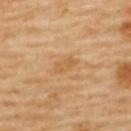Q: Was this lesion biopsied?
A: catalogued during a skin exam; not biopsied
Q: Automated lesion metrics?
A: a classifier nevus-likeness of about 0/100 and lesion-presence confidence of about 100/100
Q: What is the anatomic site?
A: the upper back
Q: What kind of image is this?
A: ~15 mm tile from a whole-body skin photo
Q: Lesion size?
A: ≈3 mm
Q: Who is the patient?
A: female, aged approximately 60